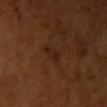Recorded during total-body skin imaging; not selected for excision or biopsy. A roughly 15 mm field-of-view crop from a total-body skin photograph. About 3 mm across. The lesion is located on the right upper arm. A female patient, about 55 years old. Captured under cross-polarized illumination.